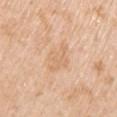Clinical impression: Captured during whole-body skin photography for melanoma surveillance; the lesion was not biopsied. Background: The lesion is located on the arm. A female subject approximately 60 years of age. A lesion tile, about 15 mm wide, cut from a 3D total-body photograph.